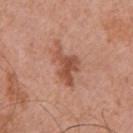No biopsy was performed on this lesion — it was imaged during a full skin examination and was not determined to be concerning. The tile uses white-light illumination. Cropped from a total-body skin-imaging series; the visible field is about 15 mm. From the chest. About 5.5 mm across. The patient is a male aged 68–72.